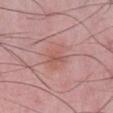This lesion was catalogued during total-body skin photography and was not selected for biopsy. Located on the chest. A region of skin cropped from a whole-body photographic capture, roughly 15 mm wide. Longest diameter approximately 4 mm. The total-body-photography lesion software estimated a lesion area of about 10 mm² and an outline eccentricity of about 0.45 (0 = round, 1 = elongated). And it measured an average lesion color of about L≈57 a*≈24 b*≈24 (CIELAB), roughly 6 lightness units darker than nearby skin, and a normalized lesion–skin contrast near 5. A male patient, aged around 50.